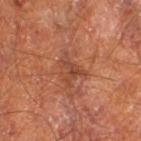| key | value |
|---|---|
| biopsy status | no biopsy performed (imaged during a skin exam) |
| automated metrics | a mean CIELAB color near L≈43 a*≈25 b*≈31 and a normalized border contrast of about 6 |
| diameter | ≈3.5 mm |
| illumination | cross-polarized illumination |
| patient | male, aged approximately 65 |
| location | the right thigh |
| image | total-body-photography crop, ~15 mm field of view |